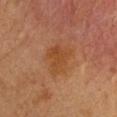Impression:
Imaged during a routine full-body skin examination; the lesion was not biopsied and no histopathology is available.
Acquisition and patient details:
The tile uses cross-polarized illumination. Automated tile analysis of the lesion measured an area of roughly 10 mm², an eccentricity of roughly 0.65, and a shape-asymmetry score of about 0.35 (0 = symmetric). It also reported an average lesion color of about L≈39 a*≈20 b*≈33 (CIELAB) and a lesion–skin lightness drop of about 6. And it measured an automated nevus-likeness rating near 0 out of 100 and a lesion-detection confidence of about 100/100. The subject is a female aged 38 to 42. A close-up tile cropped from a whole-body skin photograph, about 15 mm across. The lesion is on the arm.